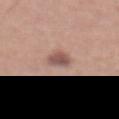Recorded during total-body skin imaging; not selected for excision or biopsy. The lesion's longest dimension is about 2.5 mm. This image is a 15 mm lesion crop taken from a total-body photograph. The lesion is on the mid back. A male patient aged around 45.